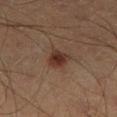A 15 mm close-up tile from a total-body photography series done for melanoma screening.
Automated tile analysis of the lesion measured an average lesion color of about L≈29 a*≈17 b*≈22 (CIELAB), about 9 CIELAB-L* units darker than the surrounding skin, and a normalized border contrast of about 9. The software also gave a border-irregularity rating of about 2.5/10.
Located on the right lower leg.
The recorded lesion diameter is about 3 mm.
A male subject approximately 40 years of age.